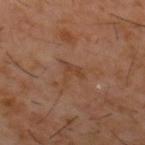Captured during whole-body skin photography for melanoma surveillance; the lesion was not biopsied. A male subject, aged 58 to 62. Captured under cross-polarized illumination. A lesion tile, about 15 mm wide, cut from a 3D total-body photograph. Located on the upper back.